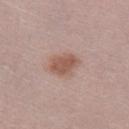biopsy status: imaged on a skin check; not biopsied | size: about 4 mm | imaging modality: total-body-photography crop, ~15 mm field of view | automated lesion analysis: a footprint of about 8.5 mm² and an outline eccentricity of about 0.65 (0 = round, 1 = elongated); a lesion color around L≈55 a*≈20 b*≈26 in CIELAB, about 10 CIELAB-L* units darker than the surrounding skin, and a normalized border contrast of about 7.5; a nevus-likeness score of about 90/100 and a lesion-detection confidence of about 100/100 | illumination: white-light illumination | patient: male, aged around 30.